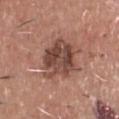Case summary:
- follow-up — no biopsy performed (imaged during a skin exam)
- imaging modality — total-body-photography crop, ~15 mm field of view
- tile lighting — white-light illumination
- diameter — about 5 mm
- site — the front of the torso
- subject — male, in their mid-40s